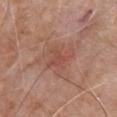workup: total-body-photography surveillance lesion; no biopsy | TBP lesion metrics: a lesion area of about 10 mm² and an eccentricity of roughly 0.65; a lesion color around L≈50 a*≈24 b*≈27 in CIELAB and a normalized border contrast of about 5; a color-variation rating of about 3.5/10 and radial color variation of about 1; a nevus-likeness score of about 0/100 | image source: 15 mm crop, total-body photography | size: about 4.5 mm | patient: male, aged around 80 | location: the chest.